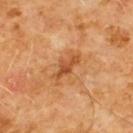{
  "biopsy_status": "not biopsied; imaged during a skin examination",
  "site": "chest",
  "patient": {
    "sex": "male",
    "age_approx": 60
  },
  "image": {
    "source": "total-body photography crop",
    "field_of_view_mm": 15
  }
}